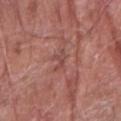The lesion was tiled from a total-body skin photograph and was not biopsied.
A 15 mm close-up tile from a total-body photography series done for melanoma screening.
From the right forearm.
An algorithmic analysis of the crop reported a lesion color around L≈47 a*≈23 b*≈24 in CIELAB and a normalized border contrast of about 5. It also reported a lesion-detection confidence of about 70/100.
The patient is a male aged around 80.
Longest diameter approximately 2.5 mm.
This is a white-light tile.